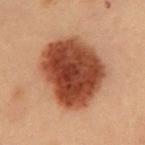| field | value |
|---|---|
| size | ≈8.5 mm |
| anatomic site | the chest |
| patient | male, approximately 55 years of age |
| image | 15 mm crop, total-body photography |
| automated lesion analysis | a border-irregularity index near 2/10, internal color variation of about 5.5 on a 0–10 scale, and radial color variation of about 1.5; a classifier nevus-likeness of about 100/100 and a lesion-detection confidence of about 100/100 |